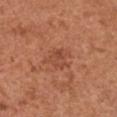{
  "biopsy_status": "not biopsied; imaged during a skin examination",
  "lesion_size": {
    "long_diameter_mm_approx": 3.5
  },
  "site": "chest",
  "image": {
    "source": "total-body photography crop",
    "field_of_view_mm": 15
  },
  "patient": {
    "sex": "female",
    "age_approx": 50
  },
  "automated_metrics": {
    "area_mm2_approx": 6.0,
    "shape_asymmetry": 0.45,
    "nevus_likeness_0_100": 0
  }
}